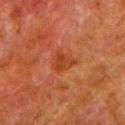No biopsy was performed on this lesion — it was imaged during a full skin examination and was not determined to be concerning.
The tile uses cross-polarized illumination.
An algorithmic analysis of the crop reported a lesion area of about 4.5 mm² and an eccentricity of roughly 0.7. The analysis additionally found a border-irregularity index near 3.5/10, internal color variation of about 2.5 on a 0–10 scale, and radial color variation of about 1. And it measured a nevus-likeness score of about 0/100 and a lesion-detection confidence of about 100/100.
A male patient, roughly 80 years of age.
Longest diameter approximately 3 mm.
The lesion is located on the left lower leg.
A 15 mm close-up extracted from a 3D total-body photography capture.